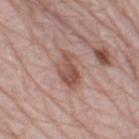<lesion>
  <biopsy_status>not biopsied; imaged during a skin examination</biopsy_status>
  <site>left thigh</site>
  <patient>
    <sex>male</sex>
    <age_approx>80</age_approx>
  </patient>
  <image>
    <source>total-body photography crop</source>
    <field_of_view_mm>15</field_of_view_mm>
  </image>
</lesion>